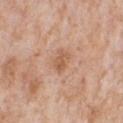workup = catalogued during a skin exam; not biopsied
imaging modality = 15 mm crop, total-body photography
patient = male, roughly 65 years of age
anatomic site = the front of the torso